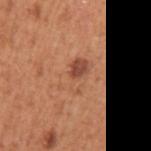This lesion was catalogued during total-body skin photography and was not selected for biopsy. Longest diameter approximately 4 mm. An algorithmic analysis of the crop reported a footprint of about 10 mm², an eccentricity of roughly 0.75, and a symmetry-axis asymmetry near 0.2. And it measured a border-irregularity rating of about 2.5/10, a color-variation rating of about 9.5/10, and a peripheral color-asymmetry measure near 4. It also reported lesion-presence confidence of about 100/100. The lesion is located on the arm. Captured under white-light illumination. The subject is a male aged approximately 55. A lesion tile, about 15 mm wide, cut from a 3D total-body photograph.